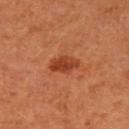notes — catalogued during a skin exam; not biopsied | lesion diameter — about 3.5 mm | image — 15 mm crop, total-body photography | lighting — cross-polarized illumination | patient — female, approximately 65 years of age | site — the right upper arm.